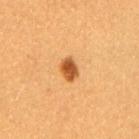The lesion was tiled from a total-body skin photograph and was not biopsied. A 15 mm close-up extracted from a 3D total-body photography capture. The lesion is on the arm. The subject is a female approximately 30 years of age.